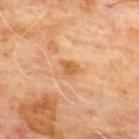Impression:
Recorded during total-body skin imaging; not selected for excision or biopsy.
Context:
About 2.5 mm across. The lesion is on the upper back. An algorithmic analysis of the crop reported a lesion area of about 3.5 mm², a shape eccentricity near 0.6, and a symmetry-axis asymmetry near 0.4. The analysis additionally found a lesion–skin lightness drop of about 9 and a lesion-to-skin contrast of about 7 (normalized; higher = more distinct). A 15 mm crop from a total-body photograph taken for skin-cancer surveillance. The subject is a male aged 63–67.